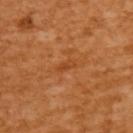workup = imaged on a skin check; not biopsied
anatomic site = the upper back
illumination = cross-polarized illumination
automated metrics = a symmetry-axis asymmetry near 0.4; roughly 7 lightness units darker than nearby skin and a normalized border contrast of about 5.5; a nevus-likeness score of about 0/100 and a lesion-detection confidence of about 100/100
imaging modality = 15 mm crop, total-body photography
subject = female, aged 53 to 57